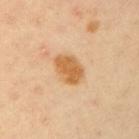Assessment: Part of a total-body skin-imaging series; this lesion was reviewed on a skin check and was not flagged for biopsy. Context: Longest diameter approximately 4 mm. From the right upper arm. A 15 mm crop from a total-body photograph taken for skin-cancer surveillance. Captured under cross-polarized illumination. The subject is a male roughly 40 years of age. The total-body-photography lesion software estimated an automated nevus-likeness rating near 75 out of 100.